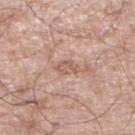Findings:
- workup — total-body-photography surveillance lesion; no biopsy
- lesion size — ~3 mm (longest diameter)
- subject — male, aged around 60
- image source — 15 mm crop, total-body photography
- location — the right lower leg